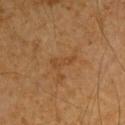Q: Was a biopsy performed?
A: total-body-photography surveillance lesion; no biopsy
Q: What are the patient's age and sex?
A: male, roughly 60 years of age
Q: What is the anatomic site?
A: the arm
Q: What kind of image is this?
A: 15 mm crop, total-body photography
Q: Illumination type?
A: cross-polarized illumination
Q: Lesion size?
A: ≈3.5 mm